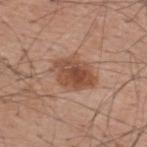Impression: Captured during whole-body skin photography for melanoma surveillance; the lesion was not biopsied. Background: On the upper back. This is a white-light tile. The patient is a male about 60 years old. Cropped from a whole-body photographic skin survey; the tile spans about 15 mm. The lesion-visualizer software estimated a footprint of about 13 mm², an outline eccentricity of about 0.7 (0 = round, 1 = elongated), and a shape-asymmetry score of about 0.2 (0 = symmetric). It also reported a mean CIELAB color near L≈49 a*≈22 b*≈30, a lesion–skin lightness drop of about 11, and a normalized lesion–skin contrast near 8.5. And it measured internal color variation of about 5 on a 0–10 scale and radial color variation of about 1.5.